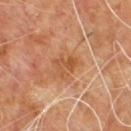The lesion was tiled from a total-body skin photograph and was not biopsied.
A 15 mm close-up extracted from a 3D total-body photography capture.
The lesion is located on the upper back.
An algorithmic analysis of the crop reported an average lesion color of about L≈51 a*≈24 b*≈37 (CIELAB) and a lesion-to-skin contrast of about 6 (normalized; higher = more distinct). The analysis additionally found a peripheral color-asymmetry measure near 1. And it measured a classifier nevus-likeness of about 0/100.
Measured at roughly 3 mm in maximum diameter.
The subject is a male approximately 60 years of age.